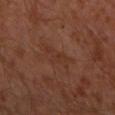biopsy_status: not biopsied; imaged during a skin examination
lighting: cross-polarized
image:
  source: total-body photography crop
  field_of_view_mm: 15
lesion_size:
  long_diameter_mm_approx: 3.5
patient:
  sex: male
  age_approx: 30
site: left upper arm